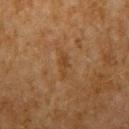  biopsy_status: not biopsied; imaged during a skin examination
  automated_metrics:
    cielab_L: 33
    cielab_a: 17
    cielab_b: 30
    vs_skin_darker_L: 5.0
    color_variation_0_10: 0.0
    nevus_likeness_0_100: 0
    lesion_detection_confidence_0_100: 95
  patient:
    sex: male
    age_approx: 65
  image:
    source: total-body photography crop
    field_of_view_mm: 15
  lesion_size:
    long_diameter_mm_approx: 3.0
  lighting: cross-polarized
  site: arm